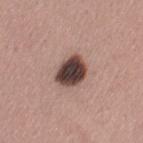Recorded during total-body skin imaging; not selected for excision or biopsy.
On the leg.
This image is a 15 mm lesion crop taken from a total-body photograph.
This is a white-light tile.
A female patient, about 30 years old.